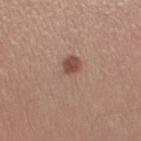follow-up — catalogued during a skin exam; not biopsied